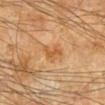Q: Was this lesion biopsied?
A: catalogued during a skin exam; not biopsied
Q: How was the tile lit?
A: cross-polarized illumination
Q: What kind of image is this?
A: ~15 mm crop, total-body skin-cancer survey
Q: What is the anatomic site?
A: the left forearm
Q: Patient demographics?
A: male, aged 68–72
Q: How large is the lesion?
A: ~2.5 mm (longest diameter)